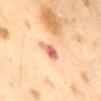An algorithmic analysis of the crop reported a shape eccentricity near 0.8 and a shape-asymmetry score of about 0.4 (0 = symmetric). The analysis additionally found a within-lesion color-variation index near 3/10 and a peripheral color-asymmetry measure near 1. Cropped from a total-body skin-imaging series; the visible field is about 15 mm. The patient is a male approximately 65 years of age. Imaged with cross-polarized lighting.